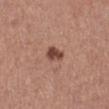Approximately 2.5 mm at its widest.
Captured under white-light illumination.
Automated image analysis of the tile measured a lesion area of about 4 mm², a shape eccentricity near 0.7, and two-axis asymmetry of about 0.4. And it measured an average lesion color of about L≈45 a*≈23 b*≈27 (CIELAB), roughly 14 lightness units darker than nearby skin, and a normalized border contrast of about 10. The software also gave a border-irregularity rating of about 3/10 and a within-lesion color-variation index near 2.5/10. The software also gave a classifier nevus-likeness of about 90/100 and a detector confidence of about 100 out of 100 that the crop contains a lesion.
A 15 mm crop from a total-body photograph taken for skin-cancer surveillance.
From the left lower leg.
The subject is a female about 60 years old.